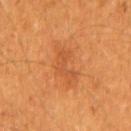Imaged during a routine full-body skin examination; the lesion was not biopsied and no histopathology is available.
Cropped from a total-body skin-imaging series; the visible field is about 15 mm.
The tile uses cross-polarized illumination.
Approximately 6 mm at its widest.
The lesion is on the back.
A male subject about 60 years old.